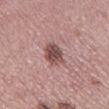{"biopsy_status": "not biopsied; imaged during a skin examination", "lighting": "white-light", "lesion_size": {"long_diameter_mm_approx": 3.5}, "patient": {"sex": "female", "age_approx": 50}, "automated_metrics": {"cielab_L": 49, "cielab_a": 21, "cielab_b": 21, "vs_skin_darker_L": 14.0, "border_irregularity_0_10": 2.5, "color_variation_0_10": 4.0, "peripheral_color_asymmetry": 1.0}, "site": "left lower leg", "image": {"source": "total-body photography crop", "field_of_view_mm": 15}}